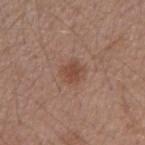Q: Was this lesion biopsied?
A: imaged on a skin check; not biopsied
Q: What is the anatomic site?
A: the right upper arm
Q: Patient demographics?
A: male, about 55 years old
Q: What is the imaging modality?
A: 15 mm crop, total-body photography